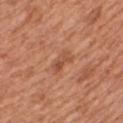Captured during whole-body skin photography for melanoma surveillance; the lesion was not biopsied.
Imaged with white-light lighting.
The lesion-visualizer software estimated a nevus-likeness score of about 0/100 and a detector confidence of about 100 out of 100 that the crop contains a lesion.
Measured at roughly 3 mm in maximum diameter.
From the chest.
A male patient, aged 63 to 67.
A lesion tile, about 15 mm wide, cut from a 3D total-body photograph.